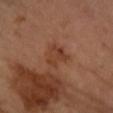<record>
<biopsy_status>not biopsied; imaged during a skin examination</biopsy_status>
<patient>
  <sex>female</sex>
  <age_approx>60</age_approx>
</patient>
<lesion_size>
  <long_diameter_mm_approx>3.0</long_diameter_mm_approx>
</lesion_size>
<site>right forearm</site>
<image>
  <source>total-body photography crop</source>
  <field_of_view_mm>15</field_of_view_mm>
</image>
</record>